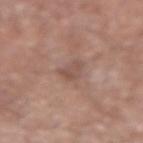Background: A region of skin cropped from a whole-body photographic capture, roughly 15 mm wide. An algorithmic analysis of the crop reported a lesion color around L≈51 a*≈19 b*≈24 in CIELAB, a lesion–skin lightness drop of about 8, and a lesion-to-skin contrast of about 5.5 (normalized; higher = more distinct). It also reported a color-variation rating of about 2.5/10 and a peripheral color-asymmetry measure near 0.5. The recorded lesion diameter is about 3 mm. The subject is a male roughly 55 years of age. This is a white-light tile. The lesion is located on the left forearm.